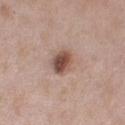Impression:
This lesion was catalogued during total-body skin photography and was not selected for biopsy.
Context:
The total-body-photography lesion software estimated a detector confidence of about 100 out of 100 that the crop contains a lesion. A roughly 15 mm field-of-view crop from a total-body skin photograph. This is a white-light tile. Located on the arm. The patient is a male approximately 55 years of age.